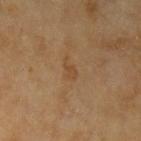<case>
  <biopsy_status>not biopsied; imaged during a skin examination</biopsy_status>
  <site>left upper arm</site>
  <lighting>cross-polarized</lighting>
  <patient>
    <sex>female</sex>
    <age_approx>60</age_approx>
  </patient>
  <lesion_size>
    <long_diameter_mm_approx>2.5</long_diameter_mm_approx>
  </lesion_size>
  <automated_metrics>
    <area_mm2_approx>3.0</area_mm2_approx>
    <shape_asymmetry>0.4</shape_asymmetry>
    <cielab_L>42</cielab_L>
    <cielab_a>16</cielab_a>
    <cielab_b>32</cielab_b>
    <vs_skin_darker_L>5.0</vs_skin_darker_L>
    <vs_skin_contrast_norm>5.0</vs_skin_contrast_norm>
    <lesion_detection_confidence_0_100>100</lesion_detection_confidence_0_100>
  </automated_metrics>
  <image>
    <source>total-body photography crop</source>
    <field_of_view_mm>15</field_of_view_mm>
  </image>
</case>